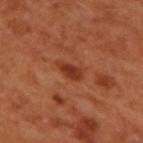The lesion was photographed on a routine skin check and not biopsied; there is no pathology result. The lesion is on the upper back. The subject is a male aged approximately 50. Longest diameter approximately 3 mm. A 15 mm crop from a total-body photograph taken for skin-cancer surveillance. The tile uses cross-polarized illumination.